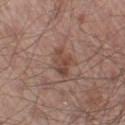A region of skin cropped from a whole-body photographic capture, roughly 15 mm wide.
A male subject roughly 55 years of age.
From the left thigh.
This is a white-light tile.